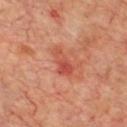Assessment:
The lesion was tiled from a total-body skin photograph and was not biopsied.
Acquisition and patient details:
This is a cross-polarized tile. The subject is a male in their 70s. Cropped from a whole-body photographic skin survey; the tile spans about 15 mm. On the chest. The lesion's longest dimension is about 4 mm.